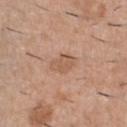follow-up: total-body-photography surveillance lesion; no biopsy | size: ≈3 mm | tile lighting: white-light | patient: male, aged 38 to 42 | anatomic site: the chest | image: 15 mm crop, total-body photography.